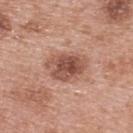The tile uses white-light illumination. Measured at roughly 5 mm in maximum diameter. The subject is a female about 60 years old. The total-body-photography lesion software estimated a mean CIELAB color near L≈51 a*≈23 b*≈27, about 13 CIELAB-L* units darker than the surrounding skin, and a normalized border contrast of about 9. It also reported border irregularity of about 2 on a 0–10 scale. The analysis additionally found a nevus-likeness score of about 75/100. From the upper back. A lesion tile, about 15 mm wide, cut from a 3D total-body photograph.